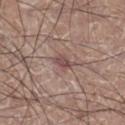{"biopsy_status": "not biopsied; imaged during a skin examination", "patient": {"sex": "male", "age_approx": 45}, "lesion_size": {"long_diameter_mm_approx": 2.5}, "lighting": "white-light", "site": "right lower leg", "automated_metrics": {"border_irregularity_0_10": 2.0, "peripheral_color_asymmetry": 0.5}, "image": {"source": "total-body photography crop", "field_of_view_mm": 15}}